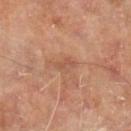Captured during whole-body skin photography for melanoma surveillance; the lesion was not biopsied. A male patient, aged approximately 70. A 15 mm crop from a total-body photograph taken for skin-cancer surveillance. The lesion is on the right lower leg.